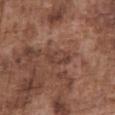• notes · imaged on a skin check; not biopsied
• acquisition · total-body-photography crop, ~15 mm field of view
• patient · male, aged around 75
• anatomic site · the abdomen